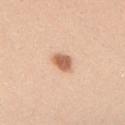follow-up: total-body-photography surveillance lesion; no biopsy | site: the back | acquisition: ~15 mm crop, total-body skin-cancer survey | subject: female, in their 20s | lighting: white-light illumination | lesion size: ≈3 mm.